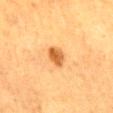No biopsy was performed on this lesion — it was imaged during a full skin examination and was not determined to be concerning.
A close-up tile cropped from a whole-body skin photograph, about 15 mm across.
Located on the mid back.
The subject is a female about 55 years old.
Measured at roughly 2.5 mm in maximum diameter.
The tile uses cross-polarized illumination.
Automated image analysis of the tile measured an automated nevus-likeness rating near 95 out of 100 and lesion-presence confidence of about 100/100.